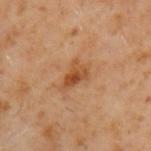Assessment: Captured during whole-body skin photography for melanoma surveillance; the lesion was not biopsied. Acquisition and patient details: A region of skin cropped from a whole-body photographic capture, roughly 15 mm wide. This is a cross-polarized tile. The subject is a male aged around 60. The lesion is located on the left upper arm.